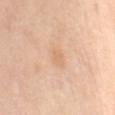The lesion was photographed on a routine skin check and not biopsied; there is no pathology result.
Captured under cross-polarized illumination.
Located on the back.
Cropped from a total-body skin-imaging series; the visible field is about 15 mm.
The subject is a female in their mid- to late 50s.
Automated image analysis of the tile measured a lesion color around L≈70 a*≈19 b*≈36 in CIELAB. The analysis additionally found an automated nevus-likeness rating near 0 out of 100 and lesion-presence confidence of about 100/100.